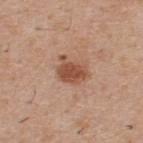workup: catalogued during a skin exam; not biopsied
patient: male, aged 58 to 62
illumination: white-light illumination
location: the upper back
image source: ~15 mm tile from a whole-body skin photo